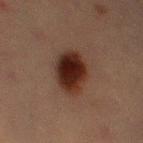This lesion was catalogued during total-body skin photography and was not selected for biopsy.
Longest diameter approximately 5 mm.
Automated tile analysis of the lesion measured a footprint of about 13 mm², an eccentricity of roughly 0.75, and a shape-asymmetry score of about 0.15 (0 = symmetric). It also reported a lesion color around L≈21 a*≈17 b*≈20 in CIELAB and a lesion–skin lightness drop of about 13. And it measured a nevus-likeness score of about 100/100 and lesion-presence confidence of about 100/100.
A region of skin cropped from a whole-body photographic capture, roughly 15 mm wide.
Located on the leg.
Imaged with cross-polarized lighting.
A female subject, in their mid-50s.